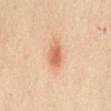illumination = cross-polarized illumination
lesion diameter = ≈3.5 mm
acquisition = 15 mm crop, total-body photography
patient = female, roughly 40 years of age
site = the chest